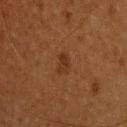Clinical summary: The tile uses cross-polarized illumination. A male subject aged 63 to 67. A region of skin cropped from a whole-body photographic capture, roughly 15 mm wide. From the head or neck. The recorded lesion diameter is about 3 mm.